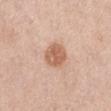Findings:
– follow-up: catalogued during a skin exam; not biopsied
– image: total-body-photography crop, ~15 mm field of view
– subject: female, aged 58 to 62
– lighting: white-light illumination
– lesion size: ~3.5 mm (longest diameter)
– site: the abdomen
– automated lesion analysis: a footprint of about 8 mm², a shape eccentricity near 0.55, and two-axis asymmetry of about 0.1; a border-irregularity index near 1/10, a color-variation rating of about 3/10, and a peripheral color-asymmetry measure near 1